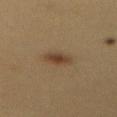Q: Was a biopsy performed?
A: catalogued during a skin exam; not biopsied